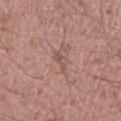The lesion was tiled from a total-body skin photograph and was not biopsied. The lesion is on the arm. This image is a 15 mm lesion crop taken from a total-body photograph. The subject is a male in their mid- to late 30s.